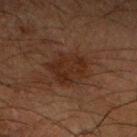The lesion was tiled from a total-body skin photograph and was not biopsied.
On the right forearm.
A male subject, aged approximately 65.
Cropped from a total-body skin-imaging series; the visible field is about 15 mm.
The total-body-photography lesion software estimated a footprint of about 14 mm², an eccentricity of roughly 0.5, and a shape-asymmetry score of about 0.2 (0 = symmetric). The analysis additionally found a lesion color around L≈22 a*≈16 b*≈22 in CIELAB, a lesion–skin lightness drop of about 6, and a normalized border contrast of about 7. The analysis additionally found a border-irregularity rating of about 2.5/10, a color-variation rating of about 2.5/10, and a peripheral color-asymmetry measure near 1. It also reported a classifier nevus-likeness of about 10/100 and a detector confidence of about 100 out of 100 that the crop contains a lesion.
Captured under cross-polarized illumination.